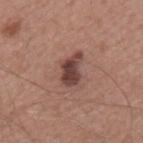Part of a total-body skin-imaging series; this lesion was reviewed on a skin check and was not flagged for biopsy. From the mid back. Imaged with white-light lighting. A male subject aged around 65. A lesion tile, about 15 mm wide, cut from a 3D total-body photograph. Approximately 4 mm at its widest.